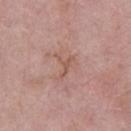workup: catalogued during a skin exam; not biopsied
lighting: white-light
location: the left lower leg
subject: female, aged 73 to 77
image source: ~15 mm tile from a whole-body skin photo